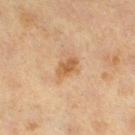The lesion was tiled from a total-body skin photograph and was not biopsied.
From the left lower leg.
A 15 mm close-up tile from a total-body photography series done for melanoma screening.
Captured under cross-polarized illumination.
Longest diameter approximately 2.5 mm.
A female patient approximately 40 years of age.